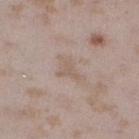Assessment:
No biopsy was performed on this lesion — it was imaged during a full skin examination and was not determined to be concerning.
Background:
Located on the left lower leg. Captured under white-light illumination. A 15 mm crop from a total-body photograph taken for skin-cancer surveillance. A female subject, in their mid-20s. Approximately 4 mm at its widest.